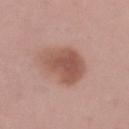No biopsy was performed on this lesion — it was imaged during a full skin examination and was not determined to be concerning. About 5.5 mm across. A roughly 15 mm field-of-view crop from a total-body skin photograph. The subject is a female aged around 45. From the left upper arm. Captured under white-light illumination.